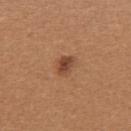Q: Was this lesion biopsied?
A: catalogued during a skin exam; not biopsied
Q: Illumination type?
A: white-light illumination
Q: Automated lesion metrics?
A: an area of roughly 4 mm², an eccentricity of roughly 0.65, and two-axis asymmetry of about 0.2; a lesion–skin lightness drop of about 11 and a normalized lesion–skin contrast near 8; lesion-presence confidence of about 100/100
Q: How was this image acquired?
A: ~15 mm tile from a whole-body skin photo
Q: Patient demographics?
A: female, in their 40s
Q: What is the anatomic site?
A: the upper back
Q: What is the lesion's diameter?
A: ≈2.5 mm